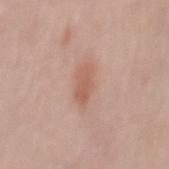Findings:
– biopsy status · imaged on a skin check; not biopsied
– lighting · white-light illumination
– patient · male, aged 48 to 52
– site · the mid back
– lesion diameter · ≈4 mm
– imaging modality · total-body-photography crop, ~15 mm field of view